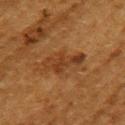Notes:
* biopsy status: total-body-photography surveillance lesion; no biopsy
* patient: female, aged 48 to 52
* body site: the arm
* image: ~15 mm crop, total-body skin-cancer survey
* tile lighting: cross-polarized